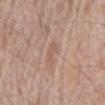{
  "biopsy_status": "not biopsied; imaged during a skin examination",
  "lighting": "white-light",
  "patient": {
    "sex": "male",
    "age_approx": 70
  },
  "lesion_size": {
    "long_diameter_mm_approx": 3.0
  },
  "site": "abdomen",
  "image": {
    "source": "total-body photography crop",
    "field_of_view_mm": 15
  }
}